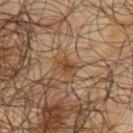Context:
A male patient, aged approximately 65. From the left upper arm. A lesion tile, about 15 mm wide, cut from a 3D total-body photograph. The lesion's longest dimension is about 2.5 mm.